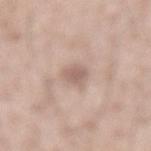patient: male, approximately 60 years of age
tile lighting: white-light illumination
anatomic site: the lower back
acquisition: ~15 mm tile from a whole-body skin photo